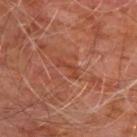Clinical impression: Recorded during total-body skin imaging; not selected for excision or biopsy. Context: A male subject approximately 60 years of age. Captured under cross-polarized illumination. On the chest. Automated image analysis of the tile measured a footprint of about 2 mm², an outline eccentricity of about 0.95 (0 = round, 1 = elongated), and two-axis asymmetry of about 0.7. The software also gave a mean CIELAB color near L≈41 a*≈28 b*≈31, about 7 CIELAB-L* units darker than the surrounding skin, and a lesion-to-skin contrast of about 5.5 (normalized; higher = more distinct). The analysis additionally found border irregularity of about 8.5 on a 0–10 scale and internal color variation of about 0 on a 0–10 scale. The lesion's longest dimension is about 3 mm. Cropped from a whole-body photographic skin survey; the tile spans about 15 mm.